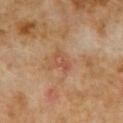A male subject about 60 years old.
Measured at roughly 2.5 mm in maximum diameter.
The lesion is on the abdomen.
A 15 mm close-up tile from a total-body photography series done for melanoma screening.